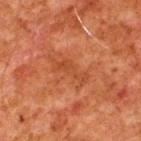<tbp_lesion>
  <biopsy_status>not biopsied; imaged during a skin examination</biopsy_status>
  <image>
    <source>total-body photography crop</source>
    <field_of_view_mm>15</field_of_view_mm>
  </image>
  <lesion_size>
    <long_diameter_mm_approx>4.5</long_diameter_mm_approx>
  </lesion_size>
  <site>upper back</site>
  <lighting>cross-polarized</lighting>
  <patient>
    <sex>male</sex>
    <age_approx>80</age_approx>
  </patient>
</tbp_lesion>